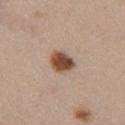biopsy status: catalogued during a skin exam; not biopsied
imaging modality: total-body-photography crop, ~15 mm field of view
body site: the left upper arm
size: about 3 mm
subject: female, aged approximately 30
illumination: white-light illumination